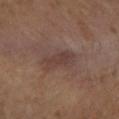Q: Was a biopsy performed?
A: no biopsy performed (imaged during a skin exam)
Q: Illumination type?
A: cross-polarized illumination
Q: Patient demographics?
A: male, roughly 65 years of age
Q: What is the lesion's diameter?
A: about 4 mm
Q: What is the imaging modality?
A: total-body-photography crop, ~15 mm field of view